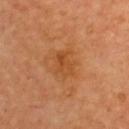Findings:
- notes — imaged on a skin check; not biopsied
- automated lesion analysis — a lesion color around L≈48 a*≈27 b*≈41 in CIELAB and a normalized border contrast of about 5.5
- patient — male, aged around 65
- tile lighting — cross-polarized
- lesion size — ≈3 mm
- acquisition — total-body-photography crop, ~15 mm field of view
- anatomic site — the back